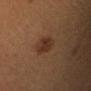Part of a total-body skin-imaging series; this lesion was reviewed on a skin check and was not flagged for biopsy. From the head or neck. This image is a 15 mm lesion crop taken from a total-body photograph. A female patient, in their 30s.